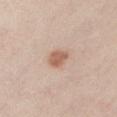Impression: Recorded during total-body skin imaging; not selected for excision or biopsy. Acquisition and patient details: A male subject approximately 25 years of age. This is a white-light tile. A 15 mm close-up tile from a total-body photography series done for melanoma screening. The lesion is on the left upper arm. Approximately 2.5 mm at its widest.